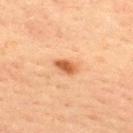Captured during whole-body skin photography for melanoma surveillance; the lesion was not biopsied. A lesion tile, about 15 mm wide, cut from a 3D total-body photograph. A male subject, aged 38 to 42. Imaged with cross-polarized lighting. The recorded lesion diameter is about 3 mm. From the upper back.